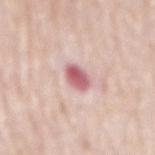Clinical impression: This lesion was catalogued during total-body skin photography and was not selected for biopsy. Clinical summary: The lesion's longest dimension is about 3 mm. The lesion is located on the back. A 15 mm close-up extracted from a 3D total-body photography capture. The subject is a male in their 80s. Automated image analysis of the tile measured a footprint of about 5.5 mm², a shape eccentricity near 0.7, and a symmetry-axis asymmetry near 0.15. It also reported a mean CIELAB color near L≈61 a*≈29 b*≈18, roughly 15 lightness units darker than nearby skin, and a lesion-to-skin contrast of about 9.5 (normalized; higher = more distinct). Captured under white-light illumination.